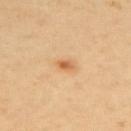Captured during whole-body skin photography for melanoma surveillance; the lesion was not biopsied.
An algorithmic analysis of the crop reported a mean CIELAB color near L≈64 a*≈24 b*≈42 and a lesion–skin lightness drop of about 11. It also reported a border-irregularity rating of about 1/10 and a color-variation rating of about 3/10. And it measured an automated nevus-likeness rating near 80 out of 100.
From the back.
The tile uses cross-polarized illumination.
A female patient, roughly 60 years of age.
A 15 mm close-up extracted from a 3D total-body photography capture.
Measured at roughly 2 mm in maximum diameter.